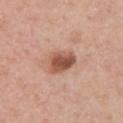  biopsy_status: not biopsied; imaged during a skin examination
  patient:
    sex: male
    age_approx: 60
  lighting: white-light
  lesion_size:
    long_diameter_mm_approx: 4.0
  automated_metrics:
    cielab_L: 53
    cielab_a: 23
    cielab_b: 30
    vs_skin_darker_L: 14.0
    vs_skin_contrast_norm: 9.5
    border_irregularity_0_10: 2.0
    color_variation_0_10: 5.0
    peripheral_color_asymmetry: 1.5
  image:
    source: total-body photography crop
    field_of_view_mm: 15
  site: chest